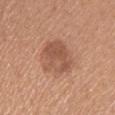Impression: Recorded during total-body skin imaging; not selected for excision or biopsy. Context: A region of skin cropped from a whole-body photographic capture, roughly 15 mm wide. On the chest. The patient is a female aged around 60.